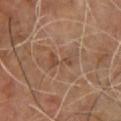{"biopsy_status": "not biopsied; imaged during a skin examination", "lighting": "cross-polarized", "patient": {"sex": "male", "age_approx": 65}, "image": {"source": "total-body photography crop", "field_of_view_mm": 15}, "site": "chest", "automated_metrics": {"vs_skin_darker_L": 6.0, "border_irregularity_0_10": 6.0, "peripheral_color_asymmetry": 0.5}}